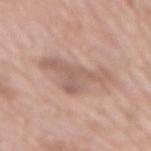<record>
<biopsy_status>not biopsied; imaged during a skin examination</biopsy_status>
<site>mid back</site>
<lighting>white-light</lighting>
<lesion_size>
  <long_diameter_mm_approx>6.5</long_diameter_mm_approx>
</lesion_size>
<image>
  <source>total-body photography crop</source>
  <field_of_view_mm>15</field_of_view_mm>
</image>
<patient>
  <sex>female</sex>
  <age_approx>75</age_approx>
</patient>
</record>